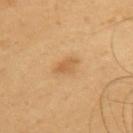  biopsy_status: not biopsied; imaged during a skin examination
  automated_metrics:
    eccentricity: 0.9
    cielab_L: 57
    cielab_a: 20
    cielab_b: 39
    vs_skin_darker_L: 8.0
    vs_skin_contrast_norm: 6.0
    peripheral_color_asymmetry: 0.5
    nevus_likeness_0_100: 60
  site: upper back
  image:
    source: total-body photography crop
    field_of_view_mm: 15
  patient:
    sex: male
    age_approx: 55
  lesion_size:
    long_diameter_mm_approx: 3.5